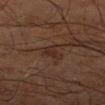Recorded during total-body skin imaging; not selected for excision or biopsy.
This is a cross-polarized tile.
Approximately 2.5 mm at its widest.
A male subject aged around 70.
On the right lower leg.
A roughly 15 mm field-of-view crop from a total-body skin photograph.
An algorithmic analysis of the crop reported a lesion color around L≈21 a*≈15 b*≈19 in CIELAB, a lesion–skin lightness drop of about 5, and a lesion-to-skin contrast of about 6 (normalized; higher = more distinct). The software also gave a border-irregularity rating of about 4.5/10, internal color variation of about 1.5 on a 0–10 scale, and a peripheral color-asymmetry measure near 0.5. The software also gave lesion-presence confidence of about 95/100.